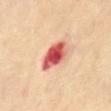Q: Was this lesion biopsied?
A: imaged on a skin check; not biopsied
Q: Patient demographics?
A: female, aged 48–52
Q: What kind of image is this?
A: 15 mm crop, total-body photography
Q: What is the anatomic site?
A: the front of the torso